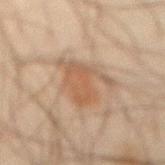Assessment:
Captured during whole-body skin photography for melanoma surveillance; the lesion was not biopsied.
Acquisition and patient details:
Measured at roughly 5 mm in maximum diameter. The lesion is on the abdomen. A 15 mm close-up tile from a total-body photography series done for melanoma screening. A male subject, about 45 years old.